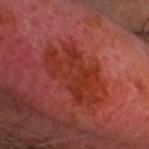<case>
  <biopsy_status>not biopsied; imaged during a skin examination</biopsy_status>
  <patient>
    <sex>male</sex>
    <age_approx>60</age_approx>
  </patient>
  <site>head or neck</site>
  <image>
    <source>total-body photography crop</source>
    <field_of_view_mm>15</field_of_view_mm>
  </image>
  <lesion_size>
    <long_diameter_mm_approx>7.5</long_diameter_mm_approx>
  </lesion_size>
</case>